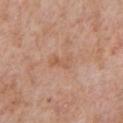Part of a total-body skin-imaging series; this lesion was reviewed on a skin check and was not flagged for biopsy. Approximately 3 mm at its widest. A male patient aged 58 to 62. The lesion is located on the chest. The lesion-visualizer software estimated a footprint of about 4.5 mm², a shape eccentricity near 0.85, and two-axis asymmetry of about 0.3. It also reported an average lesion color of about L≈58 a*≈21 b*≈32 (CIELAB) and roughly 6 lightness units darker than nearby skin. The software also gave a classifier nevus-likeness of about 0/100 and a detector confidence of about 100 out of 100 that the crop contains a lesion. The tile uses white-light illumination. A region of skin cropped from a whole-body photographic capture, roughly 15 mm wide.